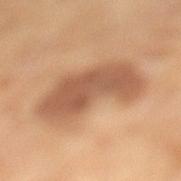Q: Is there a histopathology result?
A: imaged on a skin check; not biopsied
Q: How was this image acquired?
A: total-body-photography crop, ~15 mm field of view
Q: What is the anatomic site?
A: the left lower leg
Q: Who is the patient?
A: female, aged 68 to 72
Q: What is the lesion's diameter?
A: about 8 mm
Q: What lighting was used for the tile?
A: cross-polarized